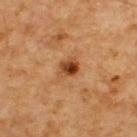Clinical impression:
No biopsy was performed on this lesion — it was imaged during a full skin examination and was not determined to be concerning.
Image and clinical context:
The lesion is on the upper back. Approximately 2.5 mm at its widest. A 15 mm crop from a total-body photograph taken for skin-cancer surveillance. A male subject aged 58 to 62.